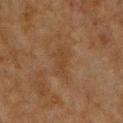Imaged during a routine full-body skin examination; the lesion was not biopsied and no histopathology is available. The lesion's longest dimension is about 4 mm. The lesion is located on the chest. Automated image analysis of the tile measured a border-irregularity rating of about 4.5/10 and a within-lesion color-variation index near 2/10. The software also gave an automated nevus-likeness rating near 0 out of 100 and a lesion-detection confidence of about 95/100. A 15 mm close-up extracted from a 3D total-body photography capture. Imaged with cross-polarized lighting. The subject is a male roughly 60 years of age.